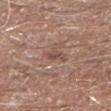Imaged during a routine full-body skin examination; the lesion was not biopsied and no histopathology is available.
The subject is a male approximately 80 years of age.
This image is a 15 mm lesion crop taken from a total-body photograph.
The lesion is located on the leg.
Automated image analysis of the tile measured a mean CIELAB color near L≈49 a*≈19 b*≈24, a lesion–skin lightness drop of about 8, and a normalized lesion–skin contrast near 6. It also reported a border-irregularity index near 3/10 and peripheral color asymmetry of about 0.5. It also reported lesion-presence confidence of about 95/100.
Longest diameter approximately 2.5 mm.